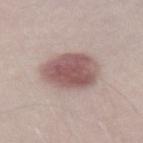{"biopsy_status": "not biopsied; imaged during a skin examination", "lighting": "white-light", "lesion_size": {"long_diameter_mm_approx": 5.5}, "image": {"source": "total-body photography crop", "field_of_view_mm": 15}, "patient": {"sex": "male", "age_approx": 30}}